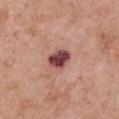Captured during whole-body skin photography for melanoma surveillance; the lesion was not biopsied.